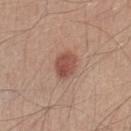  biopsy_status: not biopsied; imaged during a skin examination
  patient:
    sex: male
    age_approx: 40
  lighting: white-light
  site: left lower leg
  automated_metrics:
    nevus_likeness_0_100: 95
    lesion_detection_confidence_0_100: 100
  image:
    source: total-body photography crop
    field_of_view_mm: 15
  lesion_size:
    long_diameter_mm_approx: 3.0